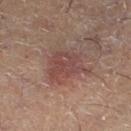<record>
  <biopsy_status>not biopsied; imaged during a skin examination</biopsy_status>
  <image>
    <source>total-body photography crop</source>
    <field_of_view_mm>15</field_of_view_mm>
  </image>
  <automated_metrics>
    <area_mm2_approx>13.0</area_mm2_approx>
    <eccentricity>0.65</eccentricity>
    <nevus_likeness_0_100>45</nevus_likeness_0_100>
    <lesion_detection_confidence_0_100>100</lesion_detection_confidence_0_100>
  </automated_metrics>
  <lighting>cross-polarized</lighting>
  <site>left lower leg</site>
  <patient>
    <sex>male</sex>
    <age_approx>50</age_approx>
  </patient>
  <lesion_size>
    <long_diameter_mm_approx>5.0</long_diameter_mm_approx>
  </lesion_size>
</record>